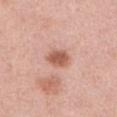notes — catalogued during a skin exam; not biopsied
automated lesion analysis — a nevus-likeness score of about 95/100
patient — female, roughly 40 years of age
image — total-body-photography crop, ~15 mm field of view
location — the abdomen
illumination — white-light
lesion diameter — about 3 mm